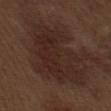{"automated_metrics": {"area_mm2_approx": 37.0, "eccentricity": 0.85, "shape_asymmetry": 0.35, "cielab_L": 25, "cielab_a": 17, "cielab_b": 21, "vs_skin_darker_L": 6.0, "vs_skin_contrast_norm": 7.0}, "lesion_size": {"long_diameter_mm_approx": 10.5}, "patient": {"sex": "male", "age_approx": 70}, "image": {"source": "total-body photography crop", "field_of_view_mm": 15}, "lighting": "white-light", "site": "left upper arm"}